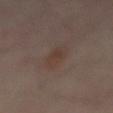Imaged during a routine full-body skin examination; the lesion was not biopsied and no histopathology is available. From the mid back. The subject is aged 53–57. Measured at roughly 4.5 mm in maximum diameter. Imaged with cross-polarized lighting. A 15 mm close-up tile from a total-body photography series done for melanoma screening.